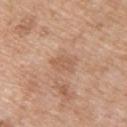A lesion tile, about 15 mm wide, cut from a 3D total-body photograph. The lesion is located on the upper back. Measured at roughly 3 mm in maximum diameter. Captured under white-light illumination. A female patient, roughly 40 years of age.